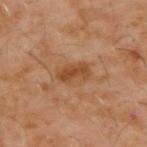{
  "biopsy_status": "not biopsied; imaged during a skin examination",
  "image": {
    "source": "total-body photography crop",
    "field_of_view_mm": 15
  },
  "site": "upper back",
  "patient": {
    "sex": "male",
    "age_approx": 60
  }
}